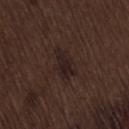The lesion was photographed on a routine skin check and not biopsied; there is no pathology result. Located on the back. A male subject, approximately 70 years of age. The tile uses white-light illumination. The lesion-visualizer software estimated an outline eccentricity of about 0.75 (0 = round, 1 = elongated) and a shape-asymmetry score of about 0.3 (0 = symmetric). It also reported border irregularity of about 3.5 on a 0–10 scale, a within-lesion color-variation index near 3.5/10, and a peripheral color-asymmetry measure near 1. The analysis additionally found lesion-presence confidence of about 100/100. A roughly 15 mm field-of-view crop from a total-body skin photograph. The lesion's longest dimension is about 4 mm.